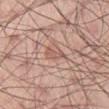Recorded during total-body skin imaging; not selected for excision or biopsy. This is a white-light tile. A male patient aged 43–47. The lesion is on the leg. Cropped from a whole-body photographic skin survey; the tile spans about 15 mm.